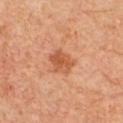No biopsy was performed on this lesion — it was imaged during a full skin examination and was not determined to be concerning. A male subject in their 60s. The lesion is located on the chest. The lesion's longest dimension is about 3 mm. The lesion-visualizer software estimated a lesion area of about 6 mm², an outline eccentricity of about 0.6 (0 = round, 1 = elongated), and a symmetry-axis asymmetry near 0.2. The software also gave border irregularity of about 2.5 on a 0–10 scale, internal color variation of about 3 on a 0–10 scale, and radial color variation of about 1. Cropped from a total-body skin-imaging series; the visible field is about 15 mm.